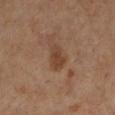Part of a total-body skin-imaging series; this lesion was reviewed on a skin check and was not flagged for biopsy. On the leg. Measured at roughly 3 mm in maximum diameter. Cropped from a whole-body photographic skin survey; the tile spans about 15 mm. Automated tile analysis of the lesion measured about 9 CIELAB-L* units darker than the surrounding skin and a normalized border contrast of about 7.5. The analysis additionally found a within-lesion color-variation index near 2.5/10 and a peripheral color-asymmetry measure near 1. It also reported an automated nevus-likeness rating near 70 out of 100. Imaged with cross-polarized lighting. A female subject aged 53–57.